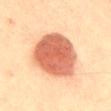Recorded during total-body skin imaging; not selected for excision or biopsy. The recorded lesion diameter is about 7 mm. The lesion is on the back. Cropped from a whole-body photographic skin survey; the tile spans about 15 mm. A male patient, roughly 40 years of age.